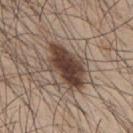Impression:
Recorded during total-body skin imaging; not selected for excision or biopsy.
Clinical summary:
A 15 mm crop from a total-body photograph taken for skin-cancer surveillance. Longest diameter approximately 6 mm. A male patient aged 43 to 47. The lesion is on the upper back. This is a white-light tile.